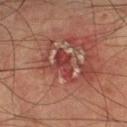biopsy status: no biopsy performed (imaged during a skin exam); image: ~15 mm tile from a whole-body skin photo; body site: the right lower leg; patient: male, aged 73 to 77.